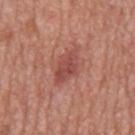<record>
  <image>
    <source>total-body photography crop</source>
    <field_of_view_mm>15</field_of_view_mm>
  </image>
  <lesion_size>
    <long_diameter_mm_approx>5.0</long_diameter_mm_approx>
  </lesion_size>
  <lighting>white-light</lighting>
  <automated_metrics>
    <area_mm2_approx>8.0</area_mm2_approx>
    <eccentricity>0.85</eccentricity>
    <border_irregularity_0_10>2.5</border_irregularity_0_10>
    <color_variation_0_10>3.0</color_variation_0_10>
    <peripheral_color_asymmetry>1.0</peripheral_color_asymmetry>
    <nevus_likeness_0_100>5</nevus_likeness_0_100>
    <lesion_detection_confidence_0_100>100</lesion_detection_confidence_0_100>
  </automated_metrics>
  <patient>
    <sex>male</sex>
    <age_approx>65</age_approx>
  </patient>
  <site>mid back</site>
</record>